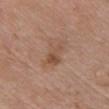The tile uses white-light illumination. On the chest. A 15 mm close-up tile from a total-body photography series done for melanoma screening. A male patient aged around 65. Approximately 4.5 mm at its widest.